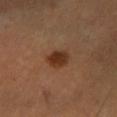{
  "biopsy_status": "not biopsied; imaged during a skin examination",
  "lighting": "cross-polarized",
  "automated_metrics": {
    "area_mm2_approx": 6.0,
    "eccentricity": 0.6,
    "shape_asymmetry": 0.15,
    "nevus_likeness_0_100": 100,
    "lesion_detection_confidence_0_100": 100
  },
  "site": "left lower leg",
  "image": {
    "source": "total-body photography crop",
    "field_of_view_mm": 15
  },
  "patient": {
    "sex": "male",
    "age_approx": 50
  },
  "lesion_size": {
    "long_diameter_mm_approx": 3.0
  }
}